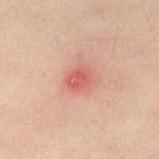  biopsy_status: not biopsied; imaged during a skin examination
  lesion_size:
    long_diameter_mm_approx: 2.5
  image:
    source: total-body photography crop
    field_of_view_mm: 15
  site: left lower leg
  lighting: cross-polarized
  automated_metrics:
    cielab_L: 46
    cielab_a: 26
    cielab_b: 24
    vs_skin_contrast_norm: 6.5
    color_variation_0_10: 1.5
    peripheral_color_asymmetry: 0.5
  patient:
    sex: female
    age_approx: 30